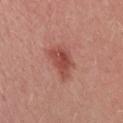Part of a total-body skin-imaging series; this lesion was reviewed on a skin check and was not flagged for biopsy. A close-up tile cropped from a whole-body skin photograph, about 15 mm across. This is a white-light tile. The lesion is located on the chest. A female patient in their mid- to late 20s.